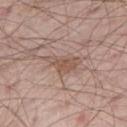Imaged during a routine full-body skin examination; the lesion was not biopsied and no histopathology is available.
A male subject in their mid- to late 60s.
This is a white-light tile.
A close-up tile cropped from a whole-body skin photograph, about 15 mm across.
Located on the right thigh.
Automated tile analysis of the lesion measured a footprint of about 6.5 mm² and two-axis asymmetry of about 0.5. The analysis additionally found a lesion color around L≈53 a*≈17 b*≈26 in CIELAB, roughly 8 lightness units darker than nearby skin, and a lesion-to-skin contrast of about 6.5 (normalized; higher = more distinct).
Longest diameter approximately 4 mm.